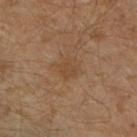The lesion was photographed on a routine skin check and not biopsied; there is no pathology result.